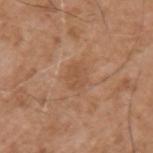Q: Is there a histopathology result?
A: no biopsy performed (imaged during a skin exam)